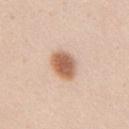Q: Was this lesion biopsied?
A: total-body-photography surveillance lesion; no biopsy
Q: How was this image acquired?
A: ~15 mm tile from a whole-body skin photo
Q: What is the anatomic site?
A: the right upper arm
Q: Patient demographics?
A: female, approximately 20 years of age